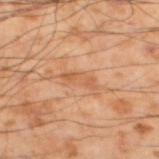  biopsy_status: not biopsied; imaged during a skin examination
  patient:
    sex: male
    age_approx: 55
  image:
    source: total-body photography crop
    field_of_view_mm: 15
  site: left lower leg
  lighting: cross-polarized
  lesion_size:
    long_diameter_mm_approx: 4.0